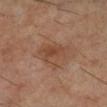workup: total-body-photography surveillance lesion; no biopsy | anatomic site: the right lower leg | lighting: cross-polarized | size: ≈4.5 mm | imaging modality: 15 mm crop, total-body photography | subject: male, aged approximately 60 | TBP lesion metrics: a border-irregularity index near 3/10, internal color variation of about 4 on a 0–10 scale, and peripheral color asymmetry of about 1.5.